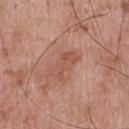{
  "biopsy_status": "not biopsied; imaged during a skin examination",
  "lighting": "white-light",
  "patient": {
    "sex": "male",
    "age_approx": 70
  },
  "automated_metrics": {
    "area_mm2_approx": 6.0,
    "eccentricity": 0.9,
    "cielab_L": 53,
    "cielab_a": 23,
    "cielab_b": 30,
    "vs_skin_darker_L": 7.0,
    "vs_skin_contrast_norm": 5.5,
    "border_irregularity_0_10": 3.5,
    "color_variation_0_10": 3.0,
    "peripheral_color_asymmetry": 1.0,
    "nevus_likeness_0_100": 0,
    "lesion_detection_confidence_0_100": 100
  },
  "lesion_size": {
    "long_diameter_mm_approx": 4.0
  },
  "site": "chest",
  "image": {
    "source": "total-body photography crop",
    "field_of_view_mm": 15
  }
}